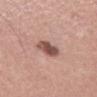Q: Was this lesion biopsied?
A: no biopsy performed (imaged during a skin exam)
Q: What kind of image is this?
A: 15 mm crop, total-body photography
Q: Where on the body is the lesion?
A: the abdomen
Q: How large is the lesion?
A: ~3 mm (longest diameter)
Q: Automated lesion metrics?
A: a shape eccentricity near 0.65; roughly 15 lightness units darker than nearby skin and a lesion-to-skin contrast of about 10 (normalized; higher = more distinct); border irregularity of about 2 on a 0–10 scale, internal color variation of about 4 on a 0–10 scale, and a peripheral color-asymmetry measure near 1; a nevus-likeness score of about 80/100
Q: What are the patient's age and sex?
A: male, in their mid- to late 50s
Q: What lighting was used for the tile?
A: white-light illumination